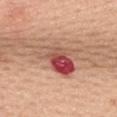notes = no biopsy performed (imaged during a skin exam)
patient = female, roughly 40 years of age
image = ~15 mm tile from a whole-body skin photo
location = the mid back